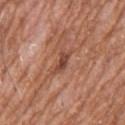Q: Is there a histopathology result?
A: no biopsy performed (imaged during a skin exam)
Q: How was the tile lit?
A: white-light
Q: What kind of image is this?
A: 15 mm crop, total-body photography
Q: How large is the lesion?
A: ≈3 mm
Q: What is the anatomic site?
A: the chest
Q: What are the patient's age and sex?
A: male, aged around 60